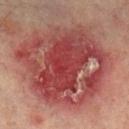No biopsy was performed on this lesion — it was imaged during a full skin examination and was not determined to be concerning. A male subject, aged 73–77. Approximately 12 mm at its widest. Automated tile analysis of the lesion measured an eccentricity of roughly 0.5 and a shape-asymmetry score of about 0.2 (0 = symmetric). The software also gave a border-irregularity rating of about 2.5/10, internal color variation of about 9.5 on a 0–10 scale, and a peripheral color-asymmetry measure near 3. From the right lower leg. This image is a 15 mm lesion crop taken from a total-body photograph. Captured under cross-polarized illumination.